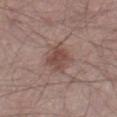biopsy status = catalogued during a skin exam; not biopsied | image source = 15 mm crop, total-body photography | subject = male, in their mid-50s | tile lighting = white-light illumination | size = ~3.5 mm (longest diameter) | body site = the right thigh.